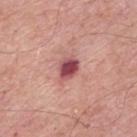Q: Was this lesion biopsied?
A: no biopsy performed (imaged during a skin exam)
Q: Patient demographics?
A: male, aged 58 to 62
Q: Illumination type?
A: white-light
Q: What is the anatomic site?
A: the upper back
Q: What kind of image is this?
A: ~15 mm crop, total-body skin-cancer survey
Q: How large is the lesion?
A: ~3 mm (longest diameter)